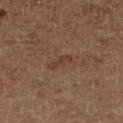Q: Was this lesion biopsied?
A: total-body-photography surveillance lesion; no biopsy